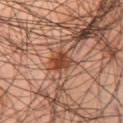Assessment: Part of a total-body skin-imaging series; this lesion was reviewed on a skin check and was not flagged for biopsy. Context: Automated image analysis of the tile measured a shape eccentricity near 0.75 and a symmetry-axis asymmetry near 0.25. It also reported a classifier nevus-likeness of about 70/100 and a detector confidence of about 100 out of 100 that the crop contains a lesion. The tile uses cross-polarized illumination. The patient is a male aged 43–47. The lesion's longest dimension is about 3.5 mm. A region of skin cropped from a whole-body photographic capture, roughly 15 mm wide. The lesion is on the right thigh.